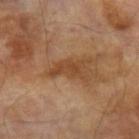site: the left forearm | lighting: cross-polarized | diameter: about 4 mm | subject: male, aged around 70 | image source: 15 mm crop, total-body photography.